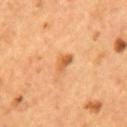{
  "biopsy_status": "not biopsied; imaged during a skin examination",
  "image": {
    "source": "total-body photography crop",
    "field_of_view_mm": 15
  },
  "site": "mid back",
  "lighting": "cross-polarized",
  "patient": {
    "sex": "male",
    "age_approx": 55
  },
  "automated_metrics": {
    "area_mm2_approx": 3.5,
    "eccentricity": 0.85,
    "shape_asymmetry": 0.35,
    "vs_skin_darker_L": 10.0
  }
}